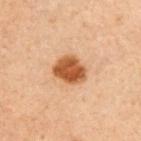Imaged during a routine full-body skin examination; the lesion was not biopsied and no histopathology is available. On the right upper arm. This image is a 15 mm lesion crop taken from a total-body photograph. Measured at roughly 3.5 mm in maximum diameter. This is a cross-polarized tile. A male patient, aged 58–62.